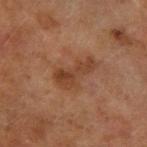Automated tile analysis of the lesion measured a classifier nevus-likeness of about 5/100 and lesion-presence confidence of about 100/100.
Captured under cross-polarized illumination.
A 15 mm crop from a total-body photograph taken for skin-cancer surveillance.
The lesion's longest dimension is about 4.5 mm.
A female patient aged 58 to 62.
The lesion is located on the left forearm.